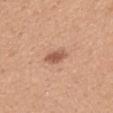Q: Is there a histopathology result?
A: catalogued during a skin exam; not biopsied
Q: How large is the lesion?
A: ≈3 mm
Q: What are the patient's age and sex?
A: female, aged around 30
Q: What is the imaging modality?
A: ~15 mm crop, total-body skin-cancer survey
Q: Where on the body is the lesion?
A: the back
Q: What lighting was used for the tile?
A: white-light illumination